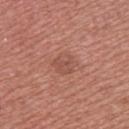No biopsy was performed on this lesion — it was imaged during a full skin examination and was not determined to be concerning. A female patient, roughly 40 years of age. The lesion is on the upper back. A 15 mm close-up tile from a total-body photography series done for melanoma screening.